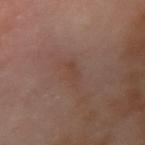Cropped from a whole-body photographic skin survey; the tile spans about 15 mm.
The patient is a male about 55 years old.
Located on the right upper arm.